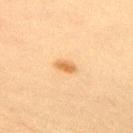Recorded during total-body skin imaging; not selected for excision or biopsy. A roughly 15 mm field-of-view crop from a total-body skin photograph. The subject is a male about 60 years old. This is a cross-polarized tile. Automated tile analysis of the lesion measured a lesion area of about 3.5 mm², an outline eccentricity of about 0.85 (0 = round, 1 = elongated), and a shape-asymmetry score of about 0.3 (0 = symmetric). And it measured an automated nevus-likeness rating near 95 out of 100 and a detector confidence of about 100 out of 100 that the crop contains a lesion. The lesion is on the right upper arm. Longest diameter approximately 2.5 mm.